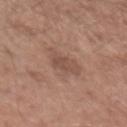Recorded during total-body skin imaging; not selected for excision or biopsy.
A male patient, roughly 50 years of age.
Longest diameter approximately 4.5 mm.
The lesion-visualizer software estimated a footprint of about 8.5 mm² and an eccentricity of roughly 0.75. The analysis additionally found a mean CIELAB color near L≈51 a*≈19 b*≈26, about 7 CIELAB-L* units darker than the surrounding skin, and a normalized lesion–skin contrast near 5.5. The analysis additionally found a border-irregularity rating of about 4/10 and a peripheral color-asymmetry measure near 1.
From the left upper arm.
Imaged with white-light lighting.
A lesion tile, about 15 mm wide, cut from a 3D total-body photograph.